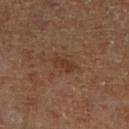Assessment: Imaged during a routine full-body skin examination; the lesion was not biopsied and no histopathology is available. Context: The recorded lesion diameter is about 2.5 mm. The lesion is located on the right lower leg. The total-body-photography lesion software estimated a lesion area of about 3.5 mm², an outline eccentricity of about 0.85 (0 = round, 1 = elongated), and a symmetry-axis asymmetry near 0.25. And it measured a border-irregularity rating of about 3/10, internal color variation of about 0.5 on a 0–10 scale, and radial color variation of about 0. A 15 mm crop from a total-body photograph taken for skin-cancer surveillance. The patient is a male aged 58 to 62.